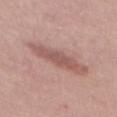  biopsy_status: not biopsied; imaged during a skin examination
  site: back
  automated_metrics:
    area_mm2_approx: 7.0
    cielab_L: 54
    cielab_a: 22
    cielab_b: 23
    vs_skin_darker_L: 10.0
    vs_skin_contrast_norm: 7.0
    border_irregularity_0_10: 3.5
    color_variation_0_10: 2.0
    peripheral_color_asymmetry: 1.0
  lighting: white-light
  patient:
    sex: female
    age_approx: 50
  image:
    source: total-body photography crop
    field_of_view_mm: 15